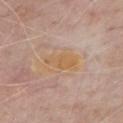Recorded during total-body skin imaging; not selected for excision or biopsy. On the chest. A male subject, aged 78 to 82. Measured at roughly 4 mm in maximum diameter. A close-up tile cropped from a whole-body skin photograph, about 15 mm across. Imaged with white-light lighting. Automated image analysis of the tile measured an average lesion color of about L≈60 a*≈18 b*≈38 (CIELAB), roughly 5 lightness units darker than nearby skin, and a normalized lesion–skin contrast near 8. The software also gave a border-irregularity rating of about 3.5/10, internal color variation of about 1.5 on a 0–10 scale, and radial color variation of about 0.5.